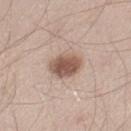<tbp_lesion>
  <biopsy_status>not biopsied; imaged during a skin examination</biopsy_status>
  <lesion_size>
    <long_diameter_mm_approx>3.5</long_diameter_mm_approx>
  </lesion_size>
  <automated_metrics>
    <area_mm2_approx>8.5</area_mm2_approx>
    <eccentricity>0.5</eccentricity>
    <shape_asymmetry>0.15</shape_asymmetry>
    <cielab_L>54</cielab_L>
    <cielab_a>18</cielab_a>
    <cielab_b>26</cielab_b>
    <vs_skin_darker_L>15.0</vs_skin_darker_L>
    <vs_skin_contrast_norm>10.0</vs_skin_contrast_norm>
    <border_irregularity_0_10>1.5</border_irregularity_0_10>
    <color_variation_0_10>4.0</color_variation_0_10>
    <peripheral_color_asymmetry>1.0</peripheral_color_asymmetry>
    <nevus_likeness_0_100>100</nevus_likeness_0_100>
    <lesion_detection_confidence_0_100>100</lesion_detection_confidence_0_100>
  </automated_metrics>
  <image>
    <source>total-body photography crop</source>
    <field_of_view_mm>15</field_of_view_mm>
  </image>
  <lighting>white-light</lighting>
  <patient>
    <sex>male</sex>
    <age_approx>35</age_approx>
  </patient>
  <site>left thigh</site>
</tbp_lesion>